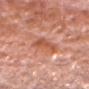About 5 mm across.
Imaged with white-light lighting.
The lesion is located on the head or neck.
An algorithmic analysis of the crop reported a lesion area of about 7 mm², an eccentricity of roughly 0.95, and a shape-asymmetry score of about 0.3 (0 = symmetric). The analysis additionally found an average lesion color of about L≈56 a*≈29 b*≈35 (CIELAB), a lesion–skin lightness drop of about 9, and a normalized lesion–skin contrast near 6.5. It also reported an automated nevus-likeness rating near 0 out of 100 and a lesion-detection confidence of about 60/100.
A roughly 15 mm field-of-view crop from a total-body skin photograph.
A male subject aged around 80.